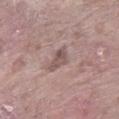The lesion was tiled from a total-body skin photograph and was not biopsied.